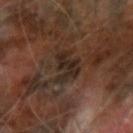{"biopsy_status": "not biopsied; imaged during a skin examination", "image": {"source": "total-body photography crop", "field_of_view_mm": 15}, "automated_metrics": {"vs_skin_darker_L": 8.0}, "site": "arm", "lighting": "cross-polarized", "patient": {"sex": "male", "age_approx": 65}}